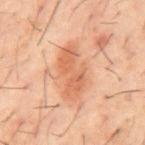biopsy_status: not biopsied; imaged during a skin examination
site: mid back
patient:
  sex: male
  age_approx: 60
lesion_size:
  long_diameter_mm_approx: 6.5
lighting: cross-polarized
image:
  source: total-body photography crop
  field_of_view_mm: 15